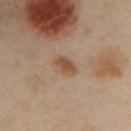Assessment:
The lesion was tiled from a total-body skin photograph and was not biopsied.
Acquisition and patient details:
This image is a 15 mm lesion crop taken from a total-body photograph. The patient is a female approximately 40 years of age. Automated image analysis of the tile measured a lesion color around L≈52 a*≈18 b*≈31 in CIELAB, about 9 CIELAB-L* units darker than the surrounding skin, and a normalized border contrast of about 7.5. It also reported an automated nevus-likeness rating near 75 out of 100 and a detector confidence of about 100 out of 100 that the crop contains a lesion. Imaged with cross-polarized lighting. About 3 mm across. The lesion is on the left arm.